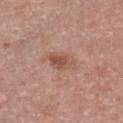Q: Is there a histopathology result?
A: imaged on a skin check; not biopsied